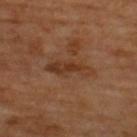Q: Was this lesion biopsied?
A: total-body-photography surveillance lesion; no biopsy
Q: What is the anatomic site?
A: the back
Q: How was this image acquired?
A: ~15 mm crop, total-body skin-cancer survey
Q: Who is the patient?
A: male, approximately 65 years of age